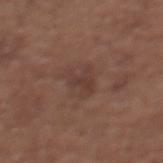biopsy_status: not biopsied; imaged during a skin examination
site: upper back
patient:
  sex: female
  age_approx: 65
image:
  source: total-body photography crop
  field_of_view_mm: 15